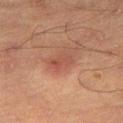| feature | finding |
|---|---|
| notes | catalogued during a skin exam; not biopsied |
| subject | male, aged 63–67 |
| anatomic site | the leg |
| acquisition | ~15 mm tile from a whole-body skin photo |